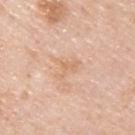Impression:
Captured during whole-body skin photography for melanoma surveillance; the lesion was not biopsied.
Clinical summary:
The tile uses white-light illumination. On the upper back. Approximately 3 mm at its widest. A 15 mm close-up tile from a total-body photography series done for melanoma screening. A male subject in their mid-40s.